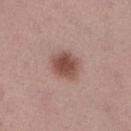Acquisition and patient details:
A female subject aged around 50. The lesion is located on the right lower leg. Cropped from a total-body skin-imaging series; the visible field is about 15 mm. Imaged with white-light lighting. Longest diameter approximately 3.5 mm.